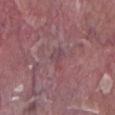Findings:
* follow-up — imaged on a skin check; not biopsied
* automated metrics — a shape eccentricity near 0.65; border irregularity of about 3.5 on a 0–10 scale and a peripheral color-asymmetry measure near 0.5; an automated nevus-likeness rating near 0 out of 100
* body site — the right lower leg
* lesion diameter — ~2.5 mm (longest diameter)
* subject — male, aged 38–42
* image source — ~15 mm tile from a whole-body skin photo